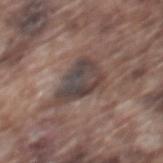biopsy status: imaged on a skin check; not biopsied
anatomic site: the mid back
TBP lesion metrics: a lesion area of about 14 mm², a shape eccentricity near 0.75, and a symmetry-axis asymmetry near 0.4; a detector confidence of about 55 out of 100 that the crop contains a lesion
lesion size: ≈5.5 mm
image source: ~15 mm tile from a whole-body skin photo
tile lighting: white-light illumination
patient: male, aged 73 to 77